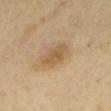biopsy_status: not biopsied; imaged during a skin examination